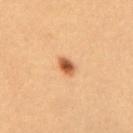biopsy status: imaged on a skin check; not biopsied
lesion diameter: ≈2.5 mm
subject: female, approximately 30 years of age
imaging modality: 15 mm crop, total-body photography
image-analysis metrics: a footprint of about 4 mm², an eccentricity of roughly 0.75, and two-axis asymmetry of about 0.25; about 15 CIELAB-L* units darker than the surrounding skin and a lesion-to-skin contrast of about 10 (normalized; higher = more distinct)
lighting: cross-polarized illumination
site: the upper back